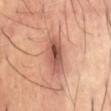subject = male, approximately 40 years of age; tile lighting = cross-polarized illumination; location = the abdomen; TBP lesion metrics = an automated nevus-likeness rating near 65 out of 100 and lesion-presence confidence of about 100/100; size = about 5.5 mm; imaging modality = total-body-photography crop, ~15 mm field of view.